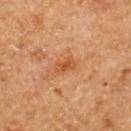TBP lesion metrics = a footprint of about 3.5 mm², an eccentricity of roughly 0.8, and a symmetry-axis asymmetry near 0.25; a within-lesion color-variation index near 3/10 and a peripheral color-asymmetry measure near 0.5; a detector confidence of about 100 out of 100 that the crop contains a lesion | imaging modality = ~15 mm crop, total-body skin-cancer survey | subject = female, aged 58 to 62 | lesion size = about 2.5 mm | location = the upper back.